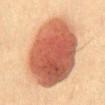Findings:
* biopsy status: total-body-photography surveillance lesion; no biopsy
* site: the mid back
* acquisition: ~15 mm crop, total-body skin-cancer survey
* patient: male, in their 40s